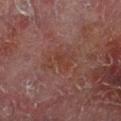Part of a total-body skin-imaging series; this lesion was reviewed on a skin check and was not flagged for biopsy. Captured under cross-polarized illumination. A male subject aged 58 to 62. On the leg. The lesion's longest dimension is about 4 mm. Cropped from a total-body skin-imaging series; the visible field is about 15 mm.